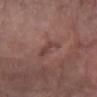Findings:
– biopsy status: imaged on a skin check; not biopsied
– patient: female, aged approximately 70
– imaging modality: ~15 mm crop, total-body skin-cancer survey
– body site: the right forearm
– diameter: ≈3 mm
– automated metrics: a lesion color around L≈42 a*≈20 b*≈22 in CIELAB, roughly 7 lightness units darker than nearby skin, and a lesion-to-skin contrast of about 6 (normalized; higher = more distinct); a classifier nevus-likeness of about 0/100 and lesion-presence confidence of about 95/100